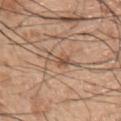workup — catalogued during a skin exam; not biopsied
TBP lesion metrics — an average lesion color of about L≈53 a*≈18 b*≈30 (CIELAB), a lesion–skin lightness drop of about 9, and a lesion-to-skin contrast of about 6.5 (normalized; higher = more distinct); a border-irregularity rating of about 5/10, a within-lesion color-variation index near 2.5/10, and peripheral color asymmetry of about 1; a nevus-likeness score of about 30/100 and lesion-presence confidence of about 95/100
tile lighting — white-light
size — about 3.5 mm
anatomic site — the chest
patient — male, roughly 45 years of age
imaging modality — total-body-photography crop, ~15 mm field of view